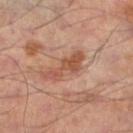| feature | finding |
|---|---|
| notes | imaged on a skin check; not biopsied |
| site | the left lower leg |
| imaging modality | ~15 mm tile from a whole-body skin photo |
| patient | male, aged around 55 |